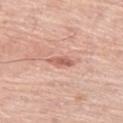The tile uses white-light illumination.
The lesion-visualizer software estimated roughly 11 lightness units darker than nearby skin and a normalized lesion–skin contrast near 7. It also reported lesion-presence confidence of about 100/100.
A male subject about 80 years old.
On the left thigh.
This image is a 15 mm lesion crop taken from a total-body photograph.